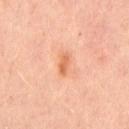Clinical impression:
The lesion was photographed on a routine skin check and not biopsied; there is no pathology result.
Context:
Captured under cross-polarized illumination. The lesion is located on the chest. Cropped from a whole-body photographic skin survey; the tile spans about 15 mm. Longest diameter approximately 2.5 mm. A male subject, in their mid-40s.